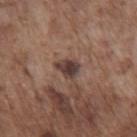| key | value |
|---|---|
| biopsy status | total-body-photography surveillance lesion; no biopsy |
| image | ~15 mm tile from a whole-body skin photo |
| subject | male, aged 73 to 77 |
| diameter | about 3 mm |
| body site | the front of the torso |
| tile lighting | white-light |